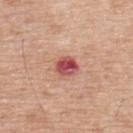notes: total-body-photography surveillance lesion; no biopsy
location: the back
patient: male, aged 68–72
automated metrics: a lesion area of about 5 mm², an outline eccentricity of about 0.65 (0 = round, 1 = elongated), and a symmetry-axis asymmetry near 0.2; an average lesion color of about L≈51 a*≈33 b*≈25 (CIELAB) and a lesion-to-skin contrast of about 11 (normalized; higher = more distinct); a border-irregularity index near 1.5/10 and internal color variation of about 7 on a 0–10 scale; a classifier nevus-likeness of about 0/100 and lesion-presence confidence of about 100/100
illumination: white-light
size: ~3 mm (longest diameter)
image: total-body-photography crop, ~15 mm field of view